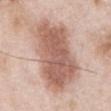Part of a total-body skin-imaging series; this lesion was reviewed on a skin check and was not flagged for biopsy.
The tile uses white-light illumination.
Measured at roughly 10 mm in maximum diameter.
The patient is a male in their mid- to late 50s.
The lesion-visualizer software estimated an eccentricity of roughly 0.85 and two-axis asymmetry of about 0.2. It also reported a lesion–skin lightness drop of about 14 and a lesion-to-skin contrast of about 9 (normalized; higher = more distinct). The analysis additionally found a border-irregularity rating of about 3.5/10 and radial color variation of about 1.5.
Located on the front of the torso.
A lesion tile, about 15 mm wide, cut from a 3D total-body photograph.